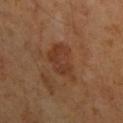Notes:
* subject · male, aged 43 to 47
* location · the left upper arm
* acquisition · ~15 mm crop, total-body skin-cancer survey